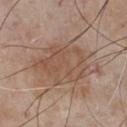Recorded during total-body skin imaging; not selected for excision or biopsy. Cropped from a whole-body photographic skin survey; the tile spans about 15 mm. Measured at roughly 6.5 mm in maximum diameter. The lesion is on the chest. Imaged with white-light lighting. Automated tile analysis of the lesion measured an eccentricity of roughly 0.7 and a symmetry-axis asymmetry near 0.3. A male patient, in their mid- to late 70s.